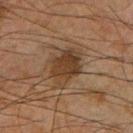Imaged during a routine full-body skin examination; the lesion was not biopsied and no histopathology is available. This is a cross-polarized tile. A 15 mm crop from a total-body photograph taken for skin-cancer surveillance. A male patient, aged 63 to 67. Located on the left thigh. An algorithmic analysis of the crop reported a border-irregularity rating of about 2.5/10 and peripheral color asymmetry of about 1. Approximately 4.5 mm at its widest.